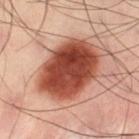| feature | finding |
|---|---|
| follow-up | catalogued during a skin exam; not biopsied |